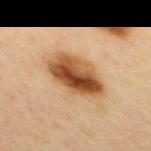{
  "biopsy_status": "not biopsied; imaged during a skin examination",
  "lighting": "cross-polarized",
  "lesion_size": {
    "long_diameter_mm_approx": 6.5
  },
  "patient": {
    "sex": "female",
    "age_approx": 45
  },
  "site": "upper back",
  "image": {
    "source": "total-body photography crop",
    "field_of_view_mm": 15
  }
}